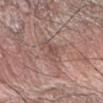Recorded during total-body skin imaging; not selected for excision or biopsy. Captured under white-light illumination. Approximately 3.5 mm at its widest. This image is a 15 mm lesion crop taken from a total-body photograph. The lesion is on the right forearm. A male patient, aged around 75.